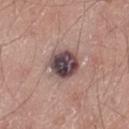The lesion was photographed on a routine skin check and not biopsied; there is no pathology result. This is a white-light tile. A male patient about 55 years old. The total-body-photography lesion software estimated a border-irregularity rating of about 1.5/10. The software also gave a classifier nevus-likeness of about 15/100 and lesion-presence confidence of about 100/100. A 15 mm close-up extracted from a 3D total-body photography capture. Located on the leg. The recorded lesion diameter is about 4 mm.